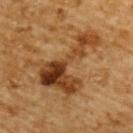Case summary:
- workup — total-body-photography surveillance lesion; no biopsy
- acquisition — ~15 mm crop, total-body skin-cancer survey
- site — the upper back
- illumination — cross-polarized illumination
- subject — male, aged 83 or older